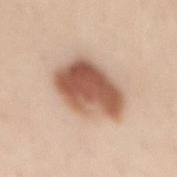Case summary:
– workup — total-body-photography surveillance lesion; no biopsy
– tile lighting — white-light
– body site — the mid back
– image source — 15 mm crop, total-body photography
– patient — female, about 40 years old
– size — ~6.5 mm (longest diameter)
– automated metrics — an area of roughly 23 mm² and an eccentricity of roughly 0.75; a nevus-likeness score of about 100/100 and a lesion-detection confidence of about 100/100